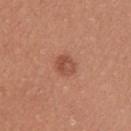Recorded during total-body skin imaging; not selected for excision or biopsy. From the left upper arm. A female patient, in their mid- to late 20s. Automated image analysis of the tile measured an eccentricity of roughly 0.55 and a symmetry-axis asymmetry near 0.15. The software also gave a lesion color around L≈50 a*≈26 b*≈31 in CIELAB, about 9 CIELAB-L* units darker than the surrounding skin, and a lesion-to-skin contrast of about 6.5 (normalized; higher = more distinct). It also reported a border-irregularity index near 1/10, a within-lesion color-variation index near 4/10, and radial color variation of about 1.5. It also reported a detector confidence of about 100 out of 100 that the crop contains a lesion. A close-up tile cropped from a whole-body skin photograph, about 15 mm across.